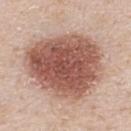Notes:
- follow-up — total-body-photography surveillance lesion; no biopsy
- size — about 9 mm
- acquisition — 15 mm crop, total-body photography
- image-analysis metrics — an outline eccentricity of about 0.55 (0 = round, 1 = elongated); about 17 CIELAB-L* units darker than the surrounding skin and a lesion-to-skin contrast of about 11 (normalized; higher = more distinct)
- anatomic site — the upper back
- subject — male, aged approximately 40